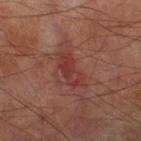Captured during whole-body skin photography for melanoma surveillance; the lesion was not biopsied.
An algorithmic analysis of the crop reported a mean CIELAB color near L≈36 a*≈27 b*≈24 and a lesion-to-skin contrast of about 6.5 (normalized; higher = more distinct). The analysis additionally found a border-irregularity rating of about 3/10, a within-lesion color-variation index near 4/10, and radial color variation of about 1.5. The software also gave a nevus-likeness score of about 5/100.
This is a cross-polarized tile.
This image is a 15 mm lesion crop taken from a total-body photograph.
On the right lower leg.
A male patient aged around 70.